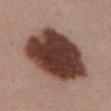workup = catalogued during a skin exam; not biopsied | location = the abdomen | imaging modality = ~15 mm tile from a whole-body skin photo | subject = female, approximately 55 years of age.